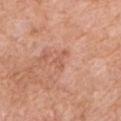Clinical impression: Recorded during total-body skin imaging; not selected for excision or biopsy. Clinical summary: Captured under white-light illumination. A male patient, about 60 years old. The lesion's longest dimension is about 2.5 mm. Located on the left upper arm. A lesion tile, about 15 mm wide, cut from a 3D total-body photograph. Automated image analysis of the tile measured a lesion area of about 2.5 mm² and a symmetry-axis asymmetry near 0.6. The software also gave an average lesion color of about L≈58 a*≈25 b*≈32 (CIELAB), a lesion–skin lightness drop of about 7, and a normalized border contrast of about 5. It also reported an automated nevus-likeness rating near 0 out of 100 and lesion-presence confidence of about 100/100.